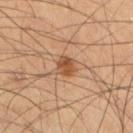<lesion>
  <biopsy_status>not biopsied; imaged during a skin examination</biopsy_status>
  <site>left thigh</site>
  <patient>
    <sex>male</sex>
    <age_approx>60</age_approx>
  </patient>
  <lesion_size>
    <long_diameter_mm_approx>2.5</long_diameter_mm_approx>
  </lesion_size>
  <lighting>cross-polarized</lighting>
  <image>
    <source>total-body photography crop</source>
    <field_of_view_mm>15</field_of_view_mm>
  </image>
</lesion>